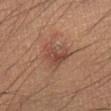From the right lower leg. This image is a 15 mm lesion crop taken from a total-body photograph. A male patient roughly 40 years of age.